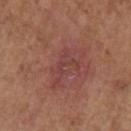Findings:
• workup · imaged on a skin check; not biopsied
• patient · female, roughly 65 years of age
• imaging modality · ~15 mm crop, total-body skin-cancer survey
• automated lesion analysis · a lesion area of about 6 mm² and a symmetry-axis asymmetry near 0.6; an average lesion color of about L≈42 a*≈25 b*≈23 (CIELAB) and a normalized lesion–skin contrast near 4.5; a within-lesion color-variation index near 1/10; a nevus-likeness score of about 0/100 and a detector confidence of about 100 out of 100 that the crop contains a lesion
• anatomic site · the left upper arm
• tile lighting · white-light